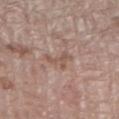Recorded during total-body skin imaging; not selected for excision or biopsy. A male patient, aged 68 to 72. From the right lower leg. Cropped from a whole-body photographic skin survey; the tile spans about 15 mm. Measured at roughly 3.5 mm in maximum diameter. Captured under white-light illumination.